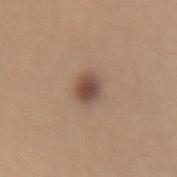Q: Was a biopsy performed?
A: catalogued during a skin exam; not biopsied
Q: What did automated image analysis measure?
A: an average lesion color of about L≈48 a*≈18 b*≈26 (CIELAB); a border-irregularity rating of about 1.5/10
Q: Lesion location?
A: the lower back
Q: Patient demographics?
A: female, in their mid- to late 30s
Q: What is the imaging modality?
A: ~15 mm tile from a whole-body skin photo